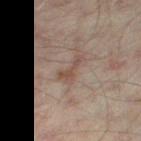Imaged during a routine full-body skin examination; the lesion was not biopsied and no histopathology is available. Cropped from a total-body skin-imaging series; the visible field is about 15 mm. The patient is a male aged 43–47. Longest diameter approximately 4 mm. From the right thigh.